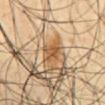notes: no biopsy performed (imaged during a skin exam)
anatomic site: the front of the torso
illumination: cross-polarized illumination
patient: male, in their 60s
TBP lesion metrics: an average lesion color of about L≈52 a*≈20 b*≈37 (CIELAB) and a normalized lesion–skin contrast near 8; an automated nevus-likeness rating near 90 out of 100
imaging modality: 15 mm crop, total-body photography
diameter: ~2.5 mm (longest diameter)